The tile uses cross-polarized illumination; a male patient, aged 38 to 42; located on the mid back; a close-up tile cropped from a whole-body skin photograph, about 15 mm across: 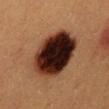• biopsy diagnosis — a melanocytic nevus